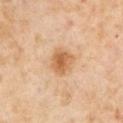Part of a total-body skin-imaging series; this lesion was reviewed on a skin check and was not flagged for biopsy.
A region of skin cropped from a whole-body photographic capture, roughly 15 mm wide.
The lesion is located on the left upper arm.
The subject is a male roughly 55 years of age.
Imaged with cross-polarized lighting.
Approximately 3 mm at its widest.